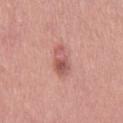Cropped from a total-body skin-imaging series; the visible field is about 15 mm.
Automated tile analysis of the lesion measured a normalized border contrast of about 7. It also reported a nevus-likeness score of about 40/100 and a detector confidence of about 100 out of 100 that the crop contains a lesion.
Captured under white-light illumination.
A female subject, aged around 40.
About 4 mm across.
The lesion is on the right thigh.